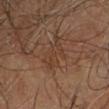This lesion was catalogued during total-body skin photography and was not selected for biopsy.
On the right leg.
A roughly 15 mm field-of-view crop from a total-body skin photograph.
The patient is a male aged approximately 60.